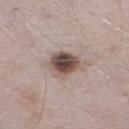This lesion was catalogued during total-body skin photography and was not selected for biopsy. A 15 mm close-up extracted from a 3D total-body photography capture. On the right lower leg. A male patient about 40 years old.